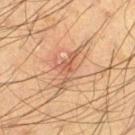| feature | finding |
|---|---|
| follow-up | catalogued during a skin exam; not biopsied |
| illumination | cross-polarized illumination |
| automated lesion analysis | border irregularity of about 8.5 on a 0–10 scale, internal color variation of about 1.5 on a 0–10 scale, and peripheral color asymmetry of about 0.5 |
| size | ~5 mm (longest diameter) |
| acquisition | ~15 mm crop, total-body skin-cancer survey |
| subject | male, in their mid-30s |
| body site | the leg |